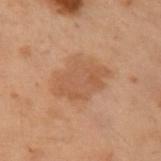Clinical impression:
No biopsy was performed on this lesion — it was imaged during a full skin examination and was not determined to be concerning.
Context:
The lesion-visualizer software estimated border irregularity of about 3 on a 0–10 scale and internal color variation of about 2.5 on a 0–10 scale. The lesion is located on the left arm. The lesion's longest dimension is about 5.5 mm. A female patient, in their mid-50s. The tile uses cross-polarized illumination. A 15 mm crop from a total-body photograph taken for skin-cancer surveillance.